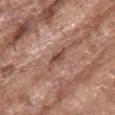follow-up = total-body-photography surveillance lesion; no biopsy | image = ~15 mm crop, total-body skin-cancer survey | subject = female, in their mid-60s | body site = the chest | illumination = white-light | size = ≈3 mm.